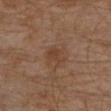A male patient, about 30 years old.
From the left lower leg.
Longest diameter approximately 2.5 mm.
The tile uses cross-polarized illumination.
A lesion tile, about 15 mm wide, cut from a 3D total-body photograph.
Automated image analysis of the tile measured a lesion color around L≈39 a*≈18 b*≈29 in CIELAB, roughly 6 lightness units darker than nearby skin, and a normalized border contrast of about 6. The analysis additionally found border irregularity of about 3.5 on a 0–10 scale and internal color variation of about 1 on a 0–10 scale.